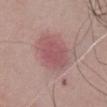Assessment: Captured during whole-body skin photography for melanoma surveillance; the lesion was not biopsied. Image and clinical context: A male patient, in their mid-80s or older. The lesion is located on the chest. Cropped from a whole-body photographic skin survey; the tile spans about 15 mm.